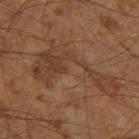• biopsy status: no biopsy performed (imaged during a skin exam)
• size: ≈10 mm
• subject: male, about 60 years old
• illumination: cross-polarized
• image source: ~15 mm crop, total-body skin-cancer survey
• body site: the left thigh